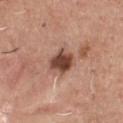biopsy_status: not biopsied; imaged during a skin examination
lesion_size:
  long_diameter_mm_approx: 3.5
patient:
  sex: male
  age_approx: 45
site: chest
automated_metrics:
  area_mm2_approx: 8.0
  eccentricity: 0.55
  shape_asymmetry: 0.2
lighting: white-light
image:
  source: total-body photography crop
  field_of_view_mm: 15